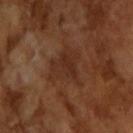Findings:
- workup: total-body-photography surveillance lesion; no biopsy
- image: ~15 mm tile from a whole-body skin photo
- patient: male, about 65 years old
- illumination: cross-polarized